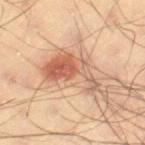Recorded during total-body skin imaging; not selected for excision or biopsy. A lesion tile, about 15 mm wide, cut from a 3D total-body photograph. From the left thigh. Captured under cross-polarized illumination. A male subject, about 60 years old.